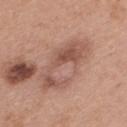Q: What is the anatomic site?
A: the upper back
Q: What kind of image is this?
A: ~15 mm crop, total-body skin-cancer survey
Q: What are the patient's age and sex?
A: female, roughly 40 years of age